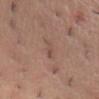The lesion was photographed on a routine skin check and not biopsied; there is no pathology result.
A female patient, approximately 30 years of age.
Imaged with white-light lighting.
Located on the abdomen.
The recorded lesion diameter is about 3 mm.
A roughly 15 mm field-of-view crop from a total-body skin photograph.